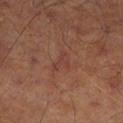Measured at roughly 3 mm in maximum diameter. The lesion is located on the leg. The patient is a male aged around 65. Automated tile analysis of the lesion measured about 5 CIELAB-L* units darker than the surrounding skin and a normalized lesion–skin contrast near 5. Cropped from a total-body skin-imaging series; the visible field is about 15 mm.